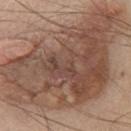Q: Was a biopsy performed?
A: imaged on a skin check; not biopsied
Q: Who is the patient?
A: male, aged 73 to 77
Q: What kind of image is this?
A: total-body-photography crop, ~15 mm field of view
Q: What is the anatomic site?
A: the chest
Q: Lesion size?
A: ~17 mm (longest diameter)